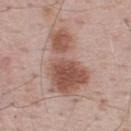A male subject in their 70s.
Longest diameter approximately 8 mm.
Located on the upper back.
Automated image analysis of the tile measured an area of roughly 25 mm², a shape eccentricity near 0.85, and a symmetry-axis asymmetry near 0.45. The analysis additionally found an average lesion color of about L≈53 a*≈21 b*≈26 (CIELAB) and a lesion-to-skin contrast of about 9 (normalized; higher = more distinct). The analysis additionally found a border-irregularity index near 6/10, a color-variation rating of about 5/10, and peripheral color asymmetry of about 2. It also reported a nevus-likeness score of about 75/100.
Captured under white-light illumination.
A roughly 15 mm field-of-view crop from a total-body skin photograph.